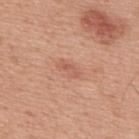Assessment:
Part of a total-body skin-imaging series; this lesion was reviewed on a skin check and was not flagged for biopsy.
Clinical summary:
Cropped from a total-body skin-imaging series; the visible field is about 15 mm. The lesion is on the upper back. An algorithmic analysis of the crop reported a footprint of about 2.5 mm², an outline eccentricity of about 0.9 (0 = round, 1 = elongated), and a shape-asymmetry score of about 0.25 (0 = symmetric). The software also gave a mean CIELAB color near L≈58 a*≈26 b*≈30, roughly 8 lightness units darker than nearby skin, and a normalized border contrast of about 5. The analysis additionally found a nevus-likeness score of about 0/100 and lesion-presence confidence of about 100/100. This is a white-light tile. Longest diameter approximately 2.5 mm. A male patient approximately 50 years of age.